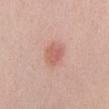Clinical impression: Recorded during total-body skin imaging; not selected for excision or biopsy. Acquisition and patient details: The lesion's longest dimension is about 3.5 mm. From the abdomen. Imaged with white-light lighting. A male subject, approximately 40 years of age. A roughly 15 mm field-of-view crop from a total-body skin photograph.